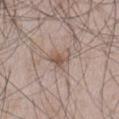A roughly 15 mm field-of-view crop from a total-body skin photograph.
Located on the abdomen.
A male patient, in their 50s.